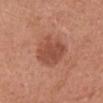{"biopsy_status": "not biopsied; imaged during a skin examination", "patient": {"sex": "female", "age_approx": 65}, "image": {"source": "total-body photography crop", "field_of_view_mm": 15}, "site": "head or neck"}